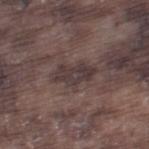Part of a total-body skin-imaging series; this lesion was reviewed on a skin check and was not flagged for biopsy.
From the left thigh.
A male subject, about 75 years old.
Measured at roughly 4.5 mm in maximum diameter.
Cropped from a total-body skin-imaging series; the visible field is about 15 mm.
Automated tile analysis of the lesion measured a shape eccentricity near 0.85. It also reported an automated nevus-likeness rating near 0 out of 100 and a detector confidence of about 55 out of 100 that the crop contains a lesion.
The tile uses white-light illumination.